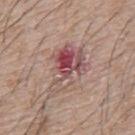• biopsy status — no biopsy performed (imaged during a skin exam)
• lesion size — ≈6.5 mm
• image-analysis metrics — an area of roughly 16 mm², an eccentricity of roughly 0.8, and a shape-asymmetry score of about 0.4 (0 = symmetric); border irregularity of about 5.5 on a 0–10 scale, a color-variation rating of about 10/10, and radial color variation of about 4.5
• patient — male, approximately 65 years of age
• illumination — white-light
• body site — the upper back
• imaging modality — 15 mm crop, total-body photography